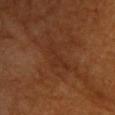Imaged during a routine full-body skin examination; the lesion was not biopsied and no histopathology is available.
The lesion is located on the front of the torso.
Cropped from a whole-body photographic skin survey; the tile spans about 15 mm.
Measured at roughly 4.5 mm in maximum diameter.
The subject is a male aged approximately 60.
Captured under cross-polarized illumination.